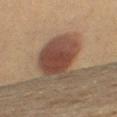No biopsy was performed on this lesion — it was imaged during a full skin examination and was not determined to be concerning.
Cropped from a whole-body photographic skin survey; the tile spans about 15 mm.
The tile uses cross-polarized illumination.
Automated tile analysis of the lesion measured a color-variation rating of about 4/10 and radial color variation of about 1. The software also gave a classifier nevus-likeness of about 100/100 and lesion-presence confidence of about 100/100.
The lesion is on the right thigh.
A female subject approximately 55 years of age.